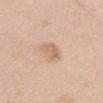Assessment:
The lesion was tiled from a total-body skin photograph and was not biopsied.
Image and clinical context:
This is a white-light tile. The recorded lesion diameter is about 3 mm. The lesion is located on the upper back. The lesion-visualizer software estimated a lesion color around L≈65 a*≈18 b*≈31 in CIELAB. And it measured border irregularity of about 2 on a 0–10 scale, a within-lesion color-variation index near 3/10, and radial color variation of about 1. The analysis additionally found an automated nevus-likeness rating near 45 out of 100 and lesion-presence confidence of about 100/100. A region of skin cropped from a whole-body photographic capture, roughly 15 mm wide. A female patient, aged 48 to 52.